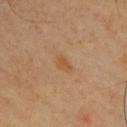Impression:
Recorded during total-body skin imaging; not selected for excision or biopsy.
Clinical summary:
A region of skin cropped from a whole-body photographic capture, roughly 15 mm wide. The patient is a male aged approximately 50. On the chest.